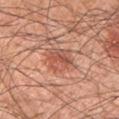- workup: total-body-photography surveillance lesion; no biopsy
- body site: the left upper arm
- subject: male, aged 58–62
- illumination: white-light
- image: ~15 mm tile from a whole-body skin photo